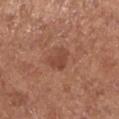{
  "biopsy_status": "not biopsied; imaged during a skin examination",
  "lighting": "white-light",
  "automated_metrics": {
    "cielab_L": 45,
    "cielab_a": 24,
    "cielab_b": 29,
    "vs_skin_darker_L": 8.0,
    "border_irregularity_0_10": 3.5,
    "peripheral_color_asymmetry": 0.5,
    "nevus_likeness_0_100": 35,
    "lesion_detection_confidence_0_100": 100
  },
  "site": "leg",
  "image": {
    "source": "total-body photography crop",
    "field_of_view_mm": 15
  },
  "patient": {
    "sex": "female",
    "age_approx": 65
  }
}